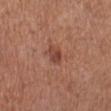{
  "biopsy_status": "not biopsied; imaged during a skin examination",
  "lesion_size": {
    "long_diameter_mm_approx": 2.5
  },
  "patient": {
    "sex": "female",
    "age_approx": 65
  },
  "image": {
    "source": "total-body photography crop",
    "field_of_view_mm": 15
  },
  "lighting": "white-light",
  "site": "leg"
}